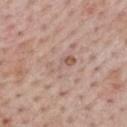  lesion_size:
    long_diameter_mm_approx: 4.0
  automated_metrics:
    border_irregularity_0_10: 7.0
    color_variation_0_10: 3.5
    peripheral_color_asymmetry: 0.5
    nevus_likeness_0_100: 0
    lesion_detection_confidence_0_100: 100
  patient:
    sex: male
    age_approx: 65
  image:
    source: total-body photography crop
    field_of_view_mm: 15
  site: back